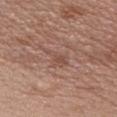| field | value |
|---|---|
| notes | total-body-photography surveillance lesion; no biopsy |
| body site | the chest |
| imaging modality | total-body-photography crop, ~15 mm field of view |
| subject | female, aged 38 to 42 |